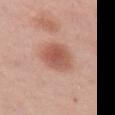{"biopsy_status": "not biopsied; imaged during a skin examination", "patient": {"sex": "female", "age_approx": 50}, "automated_metrics": {"eccentricity": 0.75, "shape_asymmetry": 0.15, "border_irregularity_0_10": 1.5, "color_variation_0_10": 4.5, "peripheral_color_asymmetry": 1.5, "nevus_likeness_0_100": 95, "lesion_detection_confidence_0_100": 100}, "image": {"source": "total-body photography crop", "field_of_view_mm": 15}, "site": "upper back"}